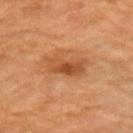Image and clinical context: Automated tile analysis of the lesion measured an average lesion color of about L≈48 a*≈25 b*≈38 (CIELAB) and about 10 CIELAB-L* units darker than the surrounding skin. And it measured a classifier nevus-likeness of about 25/100 and lesion-presence confidence of about 100/100. A roughly 15 mm field-of-view crop from a total-body skin photograph. A female subject, aged 63–67. The lesion is on the right upper arm.